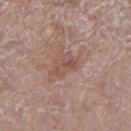<lesion>
<patient>
  <sex>female</sex>
  <age_approx>85+</age_approx>
</patient>
<image>
  <source>total-body photography crop</source>
  <field_of_view_mm>15</field_of_view_mm>
</image>
<site>right lower leg</site>
</lesion>